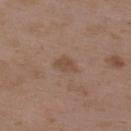Impression: This lesion was catalogued during total-body skin photography and was not selected for biopsy. Context: A roughly 15 mm field-of-view crop from a total-body skin photograph. From the right upper arm. Automated image analysis of the tile measured a border-irregularity rating of about 3/10, a within-lesion color-variation index near 1.5/10, and radial color variation of about 0.5. A female patient aged 33–37. Measured at roughly 3 mm in maximum diameter.